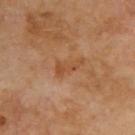Notes:
- biopsy status — catalogued during a skin exam; not biopsied
- lighting — cross-polarized
- lesion diameter — ~3.5 mm (longest diameter)
- image-analysis metrics — a lesion area of about 4.5 mm² and a shape-asymmetry score of about 0.5 (0 = symmetric); a mean CIELAB color near L≈48 a*≈23 b*≈36, a lesion–skin lightness drop of about 6, and a lesion-to-skin contrast of about 5.5 (normalized; higher = more distinct); border irregularity of about 5.5 on a 0–10 scale, a within-lesion color-variation index near 2/10, and a peripheral color-asymmetry measure near 0.5
- patient — male, roughly 70 years of age
- imaging modality — ~15 mm crop, total-body skin-cancer survey
- location — the upper back